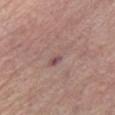Imaged during a routine full-body skin examination; the lesion was not biopsied and no histopathology is available. On the chest. A roughly 15 mm field-of-view crop from a total-body skin photograph. Captured under white-light illumination. A female patient, aged 68 to 72. About 4 mm across.